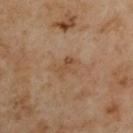acquisition=~15 mm tile from a whole-body skin photo
body site=the arm
subject=male, aged around 55
lighting=cross-polarized illumination
lesion diameter=≈2.5 mm
automated metrics=a nevus-likeness score of about 0/100 and a detector confidence of about 100 out of 100 that the crop contains a lesion